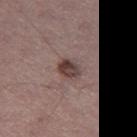workup: imaged on a skin check; not biopsied | subject: male, approximately 40 years of age | body site: the leg | tile lighting: white-light illumination | image-analysis metrics: a mean CIELAB color near L≈40 a*≈17 b*≈19, about 12 CIELAB-L* units darker than the surrounding skin, and a lesion-to-skin contrast of about 10 (normalized; higher = more distinct) | image: total-body-photography crop, ~15 mm field of view.